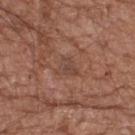Impression:
Part of a total-body skin-imaging series; this lesion was reviewed on a skin check and was not flagged for biopsy.
Image and clinical context:
On the upper back. The subject is a male approximately 75 years of age. Cropped from a total-body skin-imaging series; the visible field is about 15 mm. Imaged with white-light lighting. Automated tile analysis of the lesion measured a footprint of about 4 mm², an outline eccentricity of about 0.4 (0 = round, 1 = elongated), and a shape-asymmetry score of about 0.45 (0 = symmetric). The software also gave a lesion color around L≈44 a*≈19 b*≈26 in CIELAB, about 6 CIELAB-L* units darker than the surrounding skin, and a lesion-to-skin contrast of about 5.5 (normalized; higher = more distinct). It also reported a border-irregularity rating of about 4.5/10, internal color variation of about 2 on a 0–10 scale, and radial color variation of about 0.5. The software also gave a classifier nevus-likeness of about 0/100 and lesion-presence confidence of about 100/100. Longest diameter approximately 2.5 mm.